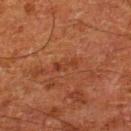This lesion was catalogued during total-body skin photography and was not selected for biopsy. The lesion is located on the leg. A 15 mm crop from a total-body photograph taken for skin-cancer surveillance. The patient is a male about 80 years old.